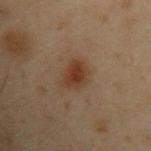Assessment:
Captured during whole-body skin photography for melanoma surveillance; the lesion was not biopsied.
Acquisition and patient details:
Captured under cross-polarized illumination. The total-body-photography lesion software estimated an eccentricity of roughly 0.75 and two-axis asymmetry of about 0.2. The software also gave an average lesion color of about L≈30 a*≈15 b*≈25 (CIELAB), roughly 8 lightness units darker than nearby skin, and a lesion-to-skin contrast of about 9 (normalized; higher = more distinct). A male subject, aged approximately 55. Cropped from a whole-body photographic skin survey; the tile spans about 15 mm. The recorded lesion diameter is about 3.5 mm. From the upper back.